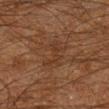notes: imaged on a skin check; not biopsied
illumination: cross-polarized illumination
image-analysis metrics: an area of roughly 4.5 mm² and two-axis asymmetry of about 0.45; a color-variation rating of about 0/10 and peripheral color asymmetry of about 0; a nevus-likeness score of about 0/100 and lesion-presence confidence of about 95/100
lesion size: ≈4 mm
body site: the leg
patient: male, aged 58 to 62
image: ~15 mm tile from a whole-body skin photo